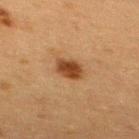Findings:
- workup · catalogued during a skin exam; not biopsied
- automated metrics · a mean CIELAB color near L≈36 a*≈19 b*≈31, roughly 12 lightness units darker than nearby skin, and a lesion-to-skin contrast of about 10.5 (normalized; higher = more distinct); a nevus-likeness score of about 100/100 and a lesion-detection confidence of about 100/100
- subject · female, roughly 55 years of age
- anatomic site · the upper back
- illumination · cross-polarized illumination
- lesion size · ~3 mm (longest diameter)
- image · total-body-photography crop, ~15 mm field of view